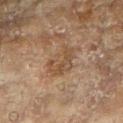biopsy status — total-body-photography surveillance lesion; no biopsy
subject — female, aged 78–82
TBP lesion metrics — a detector confidence of about 100 out of 100 that the crop contains a lesion
image source — total-body-photography crop, ~15 mm field of view
illumination — cross-polarized
anatomic site — the left arm